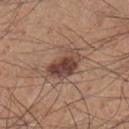The lesion was photographed on a routine skin check and not biopsied; there is no pathology result. Longest diameter approximately 4.5 mm. A male patient roughly 35 years of age. A 15 mm crop from a total-body photograph taken for skin-cancer surveillance. On the right lower leg.